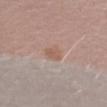| feature | finding |
|---|---|
| workup | catalogued during a skin exam; not biopsied |
| acquisition | ~15 mm crop, total-body skin-cancer survey |
| patient | female, aged approximately 50 |
| lighting | white-light illumination |
| location | the left upper arm |